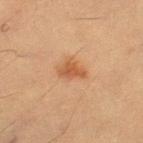No biopsy was performed on this lesion — it was imaged during a full skin examination and was not determined to be concerning. Automated image analysis of the tile measured an area of roughly 4.5 mm², a shape eccentricity near 0.8, and two-axis asymmetry of about 0.35. The analysis additionally found a border-irregularity rating of about 3.5/10, internal color variation of about 1.5 on a 0–10 scale, and a peripheral color-asymmetry measure near 0.5. The software also gave a classifier nevus-likeness of about 90/100 and a lesion-detection confidence of about 100/100. Longest diameter approximately 3 mm. Located on the left thigh. A 15 mm close-up extracted from a 3D total-body photography capture. A male patient, aged 58–62.